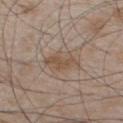follow-up — no biopsy performed (imaged during a skin exam); subject — male, aged around 50; body site — the chest; diameter — ~4.5 mm (longest diameter); image — total-body-photography crop, ~15 mm field of view; illumination — white-light.